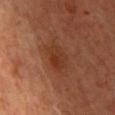This lesion was catalogued during total-body skin photography and was not selected for biopsy. A male patient roughly 60 years of age. A close-up tile cropped from a whole-body skin photograph, about 15 mm across. The lesion-visualizer software estimated an eccentricity of roughly 0.8 and a shape-asymmetry score of about 0.15 (0 = symmetric). It also reported an average lesion color of about L≈31 a*≈22 b*≈29 (CIELAB) and about 6 CIELAB-L* units darker than the surrounding skin. The analysis additionally found a nevus-likeness score of about 5/100 and a lesion-detection confidence of about 100/100. The lesion is located on the upper back. The recorded lesion diameter is about 3.5 mm.